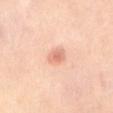Part of a total-body skin-imaging series; this lesion was reviewed on a skin check and was not flagged for biopsy. A 15 mm close-up extracted from a 3D total-body photography capture. The lesion is located on the abdomen. Automated image analysis of the tile measured an average lesion color of about L≈70 a*≈26 b*≈32 (CIELAB) and a normalized lesion–skin contrast near 6. About 2.5 mm across. A female patient aged around 40. This is a cross-polarized tile.